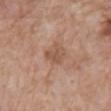Recorded during total-body skin imaging; not selected for excision or biopsy.
Cropped from a whole-body photographic skin survey; the tile spans about 15 mm.
Approximately 2.5 mm at its widest.
A male patient aged 58–62.
From the abdomen.